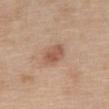{
  "biopsy_status": "not biopsied; imaged during a skin examination",
  "lesion_size": {
    "long_diameter_mm_approx": 3.5
  },
  "automated_metrics": {
    "area_mm2_approx": 6.0,
    "eccentricity": 0.85,
    "shape_asymmetry": 0.2
  },
  "patient": {
    "sex": "female",
    "age_approx": 65
  },
  "image": {
    "source": "total-body photography crop",
    "field_of_view_mm": 15
  },
  "site": "mid back",
  "lighting": "white-light"
}